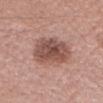Captured during whole-body skin photography for melanoma surveillance; the lesion was not biopsied.
A female subject aged 33 to 37.
The total-body-photography lesion software estimated a footprint of about 19 mm², an outline eccentricity of about 0.55 (0 = round, 1 = elongated), and two-axis asymmetry of about 0.15. The analysis additionally found a lesion color around L≈51 a*≈21 b*≈25 in CIELAB, about 13 CIELAB-L* units darker than the surrounding skin, and a normalized lesion–skin contrast near 8.5. It also reported border irregularity of about 2 on a 0–10 scale, a color-variation rating of about 5/10, and peripheral color asymmetry of about 1.5.
The lesion's longest dimension is about 5.5 mm.
On the head or neck.
A 15 mm close-up tile from a total-body photography series done for melanoma screening.
Imaged with white-light lighting.